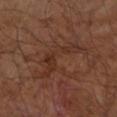The subject is a male aged approximately 65. This image is a 15 mm lesion crop taken from a total-body photograph. The lesion is on the right upper arm. Approximately 7.5 mm at its widest. The tile uses cross-polarized illumination.